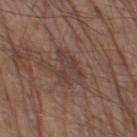patient = male, roughly 80 years of age; body site = the right thigh; image source = ~15 mm tile from a whole-body skin photo.